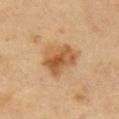{
  "biopsy_status": "not biopsied; imaged during a skin examination",
  "patient": {
    "sex": "female",
    "age_approx": 60
  },
  "image": {
    "source": "total-body photography crop",
    "field_of_view_mm": 15
  },
  "lesion_size": {
    "long_diameter_mm_approx": 4.5
  },
  "lighting": "cross-polarized",
  "site": "right upper arm"
}